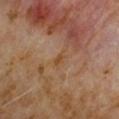Part of a total-body skin-imaging series; this lesion was reviewed on a skin check and was not flagged for biopsy.
An algorithmic analysis of the crop reported an average lesion color of about L≈44 a*≈18 b*≈33 (CIELAB), roughly 5 lightness units darker than nearby skin, and a normalized lesion–skin contrast near 6.
From the right upper arm.
A lesion tile, about 15 mm wide, cut from a 3D total-body photograph.
The recorded lesion diameter is about 2.5 mm.
A male subject in their 60s.